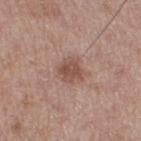automated lesion analysis = a nevus-likeness score of about 50/100 and a lesion-detection confidence of about 100/100
size = about 3 mm
subject = male, roughly 50 years of age
illumination = white-light
site = the right thigh
acquisition = ~15 mm tile from a whole-body skin photo